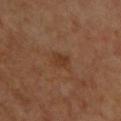follow-up: imaged on a skin check; not biopsied | illumination: cross-polarized | TBP lesion metrics: a lesion area of about 3.5 mm² and a symmetry-axis asymmetry near 0.3; a color-variation rating of about 1/10 and a peripheral color-asymmetry measure near 0.5 | location: the chest | subject: male, roughly 65 years of age | imaging modality: total-body-photography crop, ~15 mm field of view.